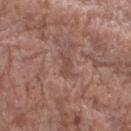{"biopsy_status": "not biopsied; imaged during a skin examination", "patient": {"sex": "male", "age_approx": 70}, "lesion_size": {"long_diameter_mm_approx": 2.5}, "site": "right forearm", "lighting": "white-light", "automated_metrics": {"cielab_L": 47, "cielab_a": 21, "cielab_b": 25, "vs_skin_darker_L": 7.0, "vs_skin_contrast_norm": 5.5}, "image": {"source": "total-body photography crop", "field_of_view_mm": 15}}